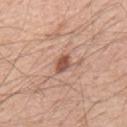Impression:
The lesion was tiled from a total-body skin photograph and was not biopsied.
Acquisition and patient details:
From the back. The lesion-visualizer software estimated an outline eccentricity of about 0.65 (0 = round, 1 = elongated). And it measured a mean CIELAB color near L≈53 a*≈23 b*≈29. And it measured border irregularity of about 1.5 on a 0–10 scale and a within-lesion color-variation index near 2.5/10. The subject is a male in their mid-50s. About 2.5 mm across. A 15 mm crop from a total-body photograph taken for skin-cancer surveillance. This is a white-light tile.